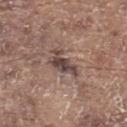Captured during whole-body skin photography for melanoma surveillance; the lesion was not biopsied. Cropped from a total-body skin-imaging series; the visible field is about 15 mm. The patient is a male aged approximately 80. The recorded lesion diameter is about 4.5 mm. From the back. This is a white-light tile.